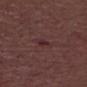Case summary:
• biopsy status — total-body-photography surveillance lesion; no biopsy
• size — ~3.5 mm (longest diameter)
• anatomic site — the head or neck
• patient — male, roughly 70 years of age
• acquisition — total-body-photography crop, ~15 mm field of view
• lighting — white-light illumination